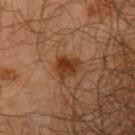{
  "biopsy_status": "not biopsied; imaged during a skin examination",
  "lighting": "cross-polarized",
  "image": {
    "source": "total-body photography crop",
    "field_of_view_mm": 15
  },
  "lesion_size": {
    "long_diameter_mm_approx": 3.5
  },
  "patient": {
    "sex": "male",
    "age_approx": 65
  },
  "site": "left upper arm"
}